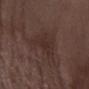| feature | finding |
|---|---|
| notes | total-body-photography surveillance lesion; no biopsy |
| acquisition | 15 mm crop, total-body photography |
| subject | male, roughly 70 years of age |
| illumination | white-light illumination |
| site | the right forearm |
| lesion diameter | ≈4.5 mm |